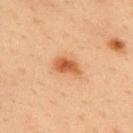<record>
  <biopsy_status>not biopsied; imaged during a skin examination</biopsy_status>
  <image>
    <source>total-body photography crop</source>
    <field_of_view_mm>15</field_of_view_mm>
  </image>
  <patient>
    <sex>male</sex>
    <age_approx>35</age_approx>
  </patient>
  <lesion_size>
    <long_diameter_mm_approx>3.5</long_diameter_mm_approx>
  </lesion_size>
  <lighting>cross-polarized</lighting>
  <site>upper back</site>
  <automated_metrics>
    <vs_skin_contrast_norm>9.0</vs_skin_contrast_norm>
  </automated_metrics>
</record>